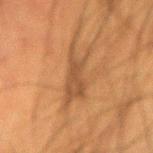The lesion was tiled from a total-body skin photograph and was not biopsied. The subject is a male aged approximately 50. A roughly 15 mm field-of-view crop from a total-body skin photograph. The lesion is located on the right upper arm.